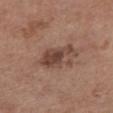{"biopsy_status": "not biopsied; imaged during a skin examination", "site": "abdomen", "patient": {"sex": "female", "age_approx": 65}, "automated_metrics": {"area_mm2_approx": 10.0, "eccentricity": 0.9, "shape_asymmetry": 0.35, "cielab_L": 44, "cielab_a": 19, "cielab_b": 25, "vs_skin_contrast_norm": 8.5, "border_irregularity_0_10": 4.5, "color_variation_0_10": 3.5, "peripheral_color_asymmetry": 1.0, "nevus_likeness_0_100": 15, "lesion_detection_confidence_0_100": 100}, "image": {"source": "total-body photography crop", "field_of_view_mm": 15}, "lighting": "white-light", "lesion_size": {"long_diameter_mm_approx": 5.5}}